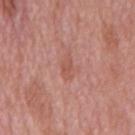Captured during whole-body skin photography for melanoma surveillance; the lesion was not biopsied. Located on the back. This is a white-light tile. Measured at roughly 3 mm in maximum diameter. A 15 mm close-up extracted from a 3D total-body photography capture. The patient is a male approximately 65 years of age.